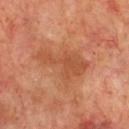Recorded during total-body skin imaging; not selected for excision or biopsy.
Automated image analysis of the tile measured an average lesion color of about L≈50 a*≈26 b*≈35 (CIELAB) and a normalized border contrast of about 5.5.
A male subject aged around 70.
Located on the front of the torso.
A 15 mm close-up tile from a total-body photography series done for melanoma screening.